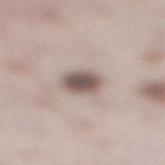Captured during whole-body skin photography for melanoma surveillance; the lesion was not biopsied. Longest diameter approximately 3.5 mm. The lesion is located on the right lower leg. Imaged with white-light lighting. A close-up tile cropped from a whole-body skin photograph, about 15 mm across. A male patient aged around 40. Automated tile analysis of the lesion measured an area of roughly 7.5 mm² and two-axis asymmetry of about 0.15. It also reported an average lesion color of about L≈54 a*≈13 b*≈19 (CIELAB), about 15 CIELAB-L* units darker than the surrounding skin, and a normalized lesion–skin contrast near 10.